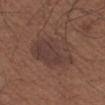| field | value |
|---|---|
| workup | catalogued during a skin exam; not biopsied |
| body site | the left upper arm |
| diameter | ~5.5 mm (longest diameter) |
| patient | male, approximately 65 years of age |
| lighting | white-light |
| acquisition | total-body-photography crop, ~15 mm field of view |